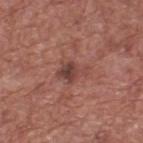No biopsy was performed on this lesion — it was imaged during a full skin examination and was not determined to be concerning. Approximately 3.5 mm at its widest. Automated tile analysis of the lesion measured a lesion-detection confidence of about 100/100. A region of skin cropped from a whole-body photographic capture, roughly 15 mm wide. From the upper back. A male subject, aged 73–77. The tile uses white-light illumination.